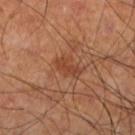Q: Was a biopsy performed?
A: imaged on a skin check; not biopsied
Q: What kind of image is this?
A: total-body-photography crop, ~15 mm field of view
Q: What are the patient's age and sex?
A: male, aged approximately 60
Q: Lesion location?
A: the left lower leg